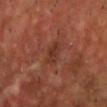Q: Was a biopsy performed?
A: imaged on a skin check; not biopsied
Q: Patient demographics?
A: male, in their mid-50s
Q: Illumination type?
A: cross-polarized illumination
Q: Lesion location?
A: the head or neck
Q: What kind of image is this?
A: 15 mm crop, total-body photography
Q: How large is the lesion?
A: ~3 mm (longest diameter)